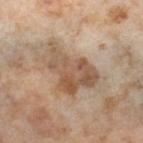Case summary:
* biopsy status: catalogued during a skin exam; not biopsied
* automated lesion analysis: a footprint of about 19 mm², a shape eccentricity near 0.75, and a shape-asymmetry score of about 0.4 (0 = symmetric)
* acquisition: 15 mm crop, total-body photography
* body site: the right thigh
* patient: female, roughly 55 years of age
* tile lighting: cross-polarized illumination